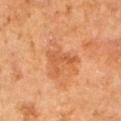No biopsy was performed on this lesion — it was imaged during a full skin examination and was not determined to be concerning.
Measured at roughly 3.5 mm in maximum diameter.
A close-up tile cropped from a whole-body skin photograph, about 15 mm across.
The lesion is on the left arm.
A male patient approximately 60 years of age.
Imaged with cross-polarized lighting.